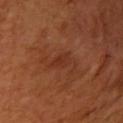This image is a 15 mm lesion crop taken from a total-body photograph.
The subject is a female aged 53–57.
The lesion is located on the right upper arm.